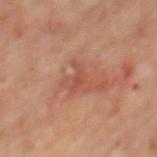Q: Was a biopsy performed?
A: imaged on a skin check; not biopsied
Q: How was the tile lit?
A: cross-polarized illumination
Q: How was this image acquired?
A: ~15 mm tile from a whole-body skin photo
Q: What are the patient's age and sex?
A: male, aged approximately 65
Q: What is the anatomic site?
A: the mid back